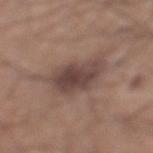Impression: Imaged during a routine full-body skin examination; the lesion was not biopsied and no histopathology is available. Clinical summary: Imaged with white-light lighting. Located on the leg. The subject is a male aged 18 to 22. Cropped from a whole-body photographic skin survey; the tile spans about 15 mm. Approximately 6 mm at its widest.